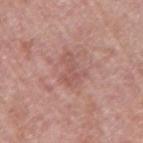{
  "biopsy_status": "not biopsied; imaged during a skin examination",
  "lesion_size": {
    "long_diameter_mm_approx": 3.5
  },
  "image": {
    "source": "total-body photography crop",
    "field_of_view_mm": 15
  },
  "lighting": "white-light",
  "patient": {
    "sex": "female",
    "age_approx": 60
  },
  "automated_metrics": {
    "area_mm2_approx": 6.5,
    "eccentricity": 0.75,
    "shape_asymmetry": 0.45,
    "cielab_L": 55,
    "cielab_a": 23,
    "cielab_b": 24,
    "border_irregularity_0_10": 6.0,
    "color_variation_0_10": 1.5,
    "peripheral_color_asymmetry": 0.5,
    "nevus_likeness_0_100": 0,
    "lesion_detection_confidence_0_100": 100
  },
  "site": "right upper arm"
}